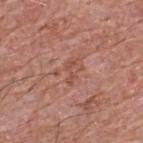Captured during whole-body skin photography for melanoma surveillance; the lesion was not biopsied. The lesion is located on the upper back. A region of skin cropped from a whole-body photographic capture, roughly 15 mm wide. A male patient, in their mid-50s.